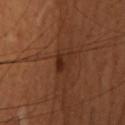No biopsy was performed on this lesion — it was imaged during a full skin examination and was not determined to be concerning. About 2.5 mm across. A female subject aged 48–52. Automated image analysis of the tile measured a lesion area of about 4.5 mm² and a shape-asymmetry score of about 0.25 (0 = symmetric). The software also gave border irregularity of about 3 on a 0–10 scale, internal color variation of about 4.5 on a 0–10 scale, and radial color variation of about 1.5. It also reported a classifier nevus-likeness of about 30/100 and a detector confidence of about 100 out of 100 that the crop contains a lesion. Cropped from a whole-body photographic skin survey; the tile spans about 15 mm. The lesion is located on the head or neck.